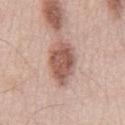Notes:
• follow-up — imaged on a skin check; not biopsied
• subject — male, aged approximately 55
• diameter — ≈6 mm
• anatomic site — the chest
• illumination — white-light
• image source — 15 mm crop, total-body photography